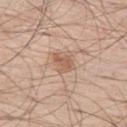notes: no biopsy performed (imaged during a skin exam)
lighting: white-light illumination
location: the leg
automated metrics: a mean CIELAB color near L≈59 a*≈20 b*≈30, about 9 CIELAB-L* units darker than the surrounding skin, and a lesion-to-skin contrast of about 6.5 (normalized; higher = more distinct); border irregularity of about 4 on a 0–10 scale, a color-variation rating of about 2/10, and peripheral color asymmetry of about 0.5; a classifier nevus-likeness of about 10/100
image: ~15 mm tile from a whole-body skin photo
patient: male, about 60 years old